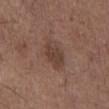{"biopsy_status": "not biopsied; imaged during a skin examination", "patient": {"sex": "male", "age_approx": 70}, "lesion_size": {"long_diameter_mm_approx": 4.5}, "site": "abdomen", "automated_metrics": {"eccentricity": 0.85, "cielab_L": 40, "cielab_a": 17, "cielab_b": 24, "vs_skin_darker_L": 8.0, "vs_skin_contrast_norm": 7.0}, "lighting": "white-light", "image": {"source": "total-body photography crop", "field_of_view_mm": 15}}